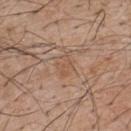The lesion was tiled from a total-body skin photograph and was not biopsied. Automated image analysis of the tile measured a lesion color around L≈54 a*≈19 b*≈31 in CIELAB, roughly 5 lightness units darker than nearby skin, and a normalized lesion–skin contrast near 4.5. The analysis additionally found a border-irregularity index near 5.5/10 and peripheral color asymmetry of about 0. Longest diameter approximately 3 mm. A male patient, in their mid-50s. The lesion is located on the upper back. Imaged with white-light lighting. Cropped from a total-body skin-imaging series; the visible field is about 15 mm.